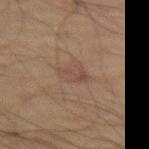* follow-up — catalogued during a skin exam; not biopsied
* subject — male, roughly 70 years of age
* size — ≈3 mm
* tile lighting — cross-polarized illumination
* imaging modality — ~15 mm crop, total-body skin-cancer survey
* site — the left thigh